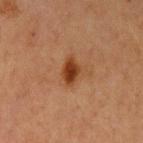The lesion was photographed on a routine skin check and not biopsied; there is no pathology result. A 15 mm crop from a total-body photograph taken for skin-cancer surveillance. The lesion is located on the left upper arm. The patient is a female aged around 40. Approximately 3 mm at its widest.